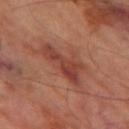The lesion was photographed on a routine skin check and not biopsied; there is no pathology result.
The lesion is located on the left thigh.
The recorded lesion diameter is about 6.5 mm.
A 15 mm close-up tile from a total-body photography series done for melanoma screening.
A male subject, about 70 years old.
Captured under cross-polarized illumination.